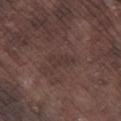The lesion was photographed on a routine skin check and not biopsied; there is no pathology result. The subject is a male roughly 75 years of age. From the right lower leg. This image is a 15 mm lesion crop taken from a total-body photograph. Approximately 3 mm at its widest.